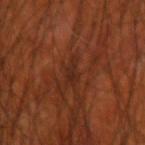Clinical summary:
Captured under cross-polarized illumination. The lesion is located on the right upper arm. A close-up tile cropped from a whole-body skin photograph, about 15 mm across. The recorded lesion diameter is about 3 mm. The subject is a male aged approximately 70.